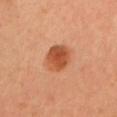Impression:
Captured during whole-body skin photography for melanoma surveillance; the lesion was not biopsied.
Image and clinical context:
Measured at roughly 4 mm in maximum diameter. A lesion tile, about 15 mm wide, cut from a 3D total-body photograph. On the chest. Imaged with cross-polarized lighting. A female subject, about 40 years old. The total-body-photography lesion software estimated a mean CIELAB color near L≈52 a*≈29 b*≈38, roughly 13 lightness units darker than nearby skin, and a lesion-to-skin contrast of about 9 (normalized; higher = more distinct). It also reported a classifier nevus-likeness of about 100/100 and a lesion-detection confidence of about 100/100.